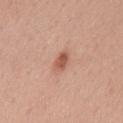follow-up = total-body-photography surveillance lesion; no biopsy
lesion size = ~2.5 mm (longest diameter)
site = the chest
acquisition = ~15 mm tile from a whole-body skin photo
illumination = white-light
automated lesion analysis = an automated nevus-likeness rating near 90 out of 100
subject = female, in their mid-30s